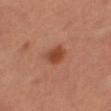Imaged during a routine full-body skin examination; the lesion was not biopsied and no histopathology is available. A region of skin cropped from a whole-body photographic capture, roughly 15 mm wide. Located on the right thigh. Measured at roughly 3 mm in maximum diameter. A female subject.